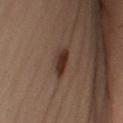The lesion was tiled from a total-body skin photograph and was not biopsied. The total-body-photography lesion software estimated a lesion color around L≈25 a*≈15 b*≈20 in CIELAB, about 9 CIELAB-L* units darker than the surrounding skin, and a normalized border contrast of about 10.5. It also reported an automated nevus-likeness rating near 95 out of 100 and a detector confidence of about 100 out of 100 that the crop contains a lesion. A male subject, roughly 50 years of age. On the left forearm. The tile uses cross-polarized illumination. Cropped from a whole-body photographic skin survey; the tile spans about 15 mm.